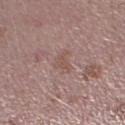- notes: catalogued during a skin exam; not biopsied
- location: the left lower leg
- illumination: white-light
- TBP lesion metrics: a mean CIELAB color near L≈52 a*≈18 b*≈23, roughly 6 lightness units darker than nearby skin, and a lesion-to-skin contrast of about 5 (normalized; higher = more distinct); a border-irregularity index near 6/10, a color-variation rating of about 1/10, and radial color variation of about 0.5; a classifier nevus-likeness of about 0/100
- image source: ~15 mm crop, total-body skin-cancer survey
- diameter: about 3 mm
- subject: male, aged 38 to 42